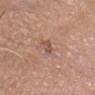notes: no biopsy performed (imaged during a skin exam) | size: ~2.5 mm (longest diameter) | anatomic site: the head or neck | patient: male, aged around 70 | tile lighting: white-light illumination | automated lesion analysis: an area of roughly 2.5 mm², an outline eccentricity of about 0.9 (0 = round, 1 = elongated), and a symmetry-axis asymmetry near 0.25; a border-irregularity rating of about 3/10, internal color variation of about 0 on a 0–10 scale, and a peripheral color-asymmetry measure near 0 | imaging modality: ~15 mm tile from a whole-body skin photo.